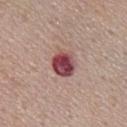follow-up: imaged on a skin check; not biopsied
tile lighting: white-light illumination
lesion diameter: ~3.5 mm (longest diameter)
body site: the chest
subject: female, about 50 years old
acquisition: ~15 mm tile from a whole-body skin photo
automated lesion analysis: a footprint of about 7.5 mm² and an eccentricity of roughly 0.5; a nevus-likeness score of about 0/100